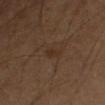Assessment:
Part of a total-body skin-imaging series; this lesion was reviewed on a skin check and was not flagged for biopsy.
Image and clinical context:
A male subject aged approximately 55. A 15 mm crop from a total-body photograph taken for skin-cancer surveillance. On the right upper arm. The tile uses cross-polarized illumination.